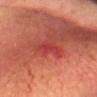Findings:
- follow-up: imaged on a skin check; not biopsied
- automated lesion analysis: an area of roughly 4.5 mm², a shape eccentricity near 0.75, and two-axis asymmetry of about 0.3; a mean CIELAB color near L≈41 a*≈40 b*≈30 and a lesion–skin lightness drop of about 6; an automated nevus-likeness rating near 0 out of 100
- location: the head or neck
- acquisition: 15 mm crop, total-body photography
- patient: male, about 65 years old